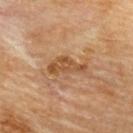  biopsy_status: not biopsied; imaged during a skin examination
  lighting: cross-polarized
  patient:
    sex: male
    age_approx: 85+
  site: upper back
  image:
    source: total-body photography crop
    field_of_view_mm: 15